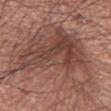Q: Was a biopsy performed?
A: catalogued during a skin exam; not biopsied
Q: Who is the patient?
A: male, about 60 years old
Q: How was this image acquired?
A: total-body-photography crop, ~15 mm field of view
Q: Where on the body is the lesion?
A: the leg